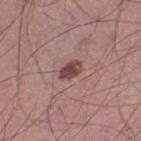• biopsy status · catalogued during a skin exam; not biopsied
• subject · male, aged 43–47
• site · the leg
• imaging modality · total-body-photography crop, ~15 mm field of view